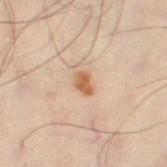Clinical impression:
This lesion was catalogued during total-body skin photography and was not selected for biopsy.